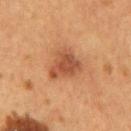{
  "biopsy_status": "not biopsied; imaged during a skin examination",
  "automated_metrics": {
    "cielab_L": 47,
    "cielab_a": 25,
    "cielab_b": 33,
    "vs_skin_darker_L": 11.0,
    "vs_skin_contrast_norm": 8.0,
    "border_irregularity_0_10": 3.5,
    "color_variation_0_10": 3.0,
    "peripheral_color_asymmetry": 1.0
  },
  "lesion_size": {
    "long_diameter_mm_approx": 4.0
  },
  "site": "mid back",
  "lighting": "cross-polarized",
  "patient": {
    "sex": "male",
    "age_approx": 55
  },
  "image": {
    "source": "total-body photography crop",
    "field_of_view_mm": 15
  }
}